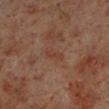Q: Is there a histopathology result?
A: no biopsy performed (imaged during a skin exam)
Q: How was this image acquired?
A: total-body-photography crop, ~15 mm field of view
Q: What is the anatomic site?
A: the left lower leg
Q: What are the patient's age and sex?
A: male, about 60 years old